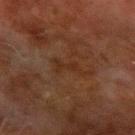Imaged during a routine full-body skin examination; the lesion was not biopsied and no histopathology is available. A region of skin cropped from a whole-body photographic capture, roughly 15 mm wide. The lesion's longest dimension is about 3.5 mm. Automated image analysis of the tile measured an average lesion color of about L≈24 a*≈17 b*≈25 (CIELAB), about 4 CIELAB-L* units darker than the surrounding skin, and a lesion-to-skin contrast of about 5 (normalized; higher = more distinct). The software also gave a border-irregularity rating of about 6/10, internal color variation of about 0 on a 0–10 scale, and radial color variation of about 0. The software also gave a classifier nevus-likeness of about 0/100 and a detector confidence of about 100 out of 100 that the crop contains a lesion. The patient is a male aged 68–72. On the left forearm.